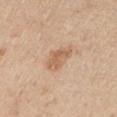Part of a total-body skin-imaging series; this lesion was reviewed on a skin check and was not flagged for biopsy.
This image is a 15 mm lesion crop taken from a total-body photograph.
The lesion is on the right upper arm.
A male patient roughly 75 years of age.